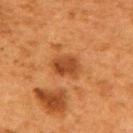Clinical impression:
Imaged during a routine full-body skin examination; the lesion was not biopsied and no histopathology is available.
Acquisition and patient details:
The lesion-visualizer software estimated an area of roughly 6.5 mm² and a shape-asymmetry score of about 0.2 (0 = symmetric). It also reported a lesion–skin lightness drop of about 10 and a lesion-to-skin contrast of about 8 (normalized; higher = more distinct). The software also gave a classifier nevus-likeness of about 45/100 and lesion-presence confidence of about 100/100. Imaged with cross-polarized lighting. The lesion's longest dimension is about 3 mm. A female subject aged 48 to 52. On the upper back. A close-up tile cropped from a whole-body skin photograph, about 15 mm across.